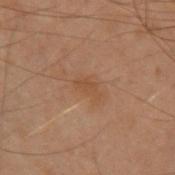Q: Is there a histopathology result?
A: no biopsy performed (imaged during a skin exam)
Q: Patient demographics?
A: male, roughly 50 years of age
Q: What is the imaging modality?
A: ~15 mm tile from a whole-body skin photo
Q: Where on the body is the lesion?
A: the left forearm